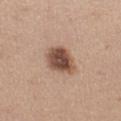Captured during whole-body skin photography for melanoma surveillance; the lesion was not biopsied. About 4 mm across. Captured under white-light illumination. A region of skin cropped from a whole-body photographic capture, roughly 15 mm wide. Located on the left thigh. A female subject, roughly 45 years of age. The lesion-visualizer software estimated a footprint of about 9.5 mm², a shape eccentricity near 0.6, and two-axis asymmetry of about 0.25. It also reported a within-lesion color-variation index near 5.5/10.